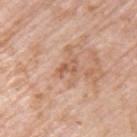Q: Is there a histopathology result?
A: imaged on a skin check; not biopsied
Q: Lesion size?
A: ~2.5 mm (longest diameter)
Q: Illumination type?
A: white-light
Q: How was this image acquired?
A: ~15 mm crop, total-body skin-cancer survey
Q: What are the patient's age and sex?
A: male, aged around 60
Q: Where on the body is the lesion?
A: the left upper arm
Q: What did automated image analysis measure?
A: an area of roughly 3 mm², an outline eccentricity of about 0.8 (0 = round, 1 = elongated), and a shape-asymmetry score of about 0.4 (0 = symmetric); a mean CIELAB color near L≈58 a*≈22 b*≈32, roughly 9 lightness units darker than nearby skin, and a lesion-to-skin contrast of about 6 (normalized; higher = more distinct); a classifier nevus-likeness of about 0/100 and a lesion-detection confidence of about 100/100